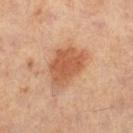{
  "biopsy_status": "not biopsied; imaged during a skin examination",
  "patient": {
    "sex": "male",
    "age_approx": 60
  },
  "automated_metrics": {
    "nevus_likeness_0_100": 100,
    "lesion_detection_confidence_0_100": 100
  },
  "image": {
    "source": "total-body photography crop",
    "field_of_view_mm": 15
  },
  "site": "left thigh"
}